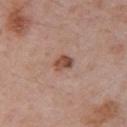{
  "image": {
    "source": "total-body photography crop",
    "field_of_view_mm": 15
  },
  "patient": {
    "sex": "male",
    "age_approx": 55
  },
  "site": "arm"
}